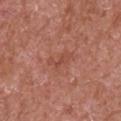workup — total-body-photography surveillance lesion; no biopsy
subject — male, in their mid- to late 60s
body site — the right upper arm
acquisition — ~15 mm tile from a whole-body skin photo